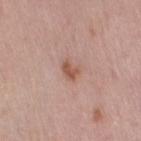notes: no biopsy performed (imaged during a skin exam)
patient: female, aged around 50
body site: the right thigh
acquisition: ~15 mm crop, total-body skin-cancer survey
TBP lesion metrics: a footprint of about 4 mm², an eccentricity of roughly 0.7, and a symmetry-axis asymmetry near 0.3; a classifier nevus-likeness of about 65/100 and a lesion-detection confidence of about 100/100
illumination: white-light
lesion size: ≈3 mm